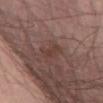Recorded during total-body skin imaging; not selected for excision or biopsy. Automated tile analysis of the lesion measured an average lesion color of about L≈39 a*≈19 b*≈22 (CIELAB) and a normalized border contrast of about 5.5. And it measured a border-irregularity index near 3.5/10, internal color variation of about 1.5 on a 0–10 scale, and peripheral color asymmetry of about 0.5. It also reported a classifier nevus-likeness of about 0/100 and lesion-presence confidence of about 100/100. Approximately 2.5 mm at its widest. The lesion is on the abdomen. This is a white-light tile. A male patient, roughly 75 years of age. A lesion tile, about 15 mm wide, cut from a 3D total-body photograph.